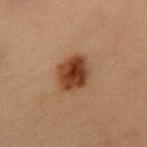Assessment: Recorded during total-body skin imaging; not selected for excision or biopsy. Acquisition and patient details: A male subject about 55 years old. A 15 mm close-up tile from a total-body photography series done for melanoma screening. The recorded lesion diameter is about 4 mm. This is a cross-polarized tile. On the abdomen.